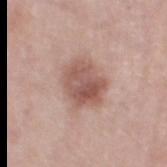Notes:
• biopsy status · catalogued during a skin exam; not biopsied
• image source · ~15 mm crop, total-body skin-cancer survey
• lighting · white-light illumination
• anatomic site · the left thigh
• subject · female, aged approximately 40
• size · ~5 mm (longest diameter)